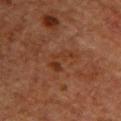biopsy status = no biopsy performed (imaged during a skin exam); subject = female, approximately 70 years of age; anatomic site = the upper back; image = total-body-photography crop, ~15 mm field of view.